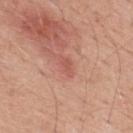Assessment: Recorded during total-body skin imaging; not selected for excision or biopsy. Clinical summary: The lesion is located on the upper back. An algorithmic analysis of the crop reported an average lesion color of about L≈55 a*≈27 b*≈28 (CIELAB) and a normalized border contrast of about 5. The analysis additionally found a nevus-likeness score of about 0/100 and a detector confidence of about 100 out of 100 that the crop contains a lesion. The subject is a male aged 53–57. The tile uses white-light illumination. A region of skin cropped from a whole-body photographic capture, roughly 15 mm wide. Measured at roughly 2.5 mm in maximum diameter.